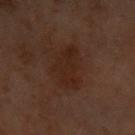The lesion was tiled from a total-body skin photograph and was not biopsied. From the right arm. A 15 mm crop from a total-body photograph taken for skin-cancer surveillance. Longest diameter approximately 5.5 mm. Imaged with cross-polarized lighting. The subject is a female aged 58–62.